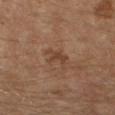Part of a total-body skin-imaging series; this lesion was reviewed on a skin check and was not flagged for biopsy. Measured at roughly 3.5 mm in maximum diameter. A female patient, aged 58–62. Imaged with cross-polarized lighting. A 15 mm close-up extracted from a 3D total-body photography capture. Located on the right forearm.